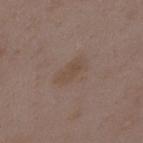Findings:
- notes · imaged on a skin check; not biopsied
- tile lighting · white-light illumination
- image · ~15 mm crop, total-body skin-cancer survey
- anatomic site · the upper back
- subject · female, aged 33–37
- diameter · about 4 mm